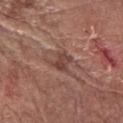Clinical impression:
This lesion was catalogued during total-body skin photography and was not selected for biopsy.
Clinical summary:
A male patient, in their mid- to late 70s. The lesion is on the right forearm. The recorded lesion diameter is about 3 mm. Imaged with white-light lighting. A 15 mm close-up extracted from a 3D total-body photography capture. An algorithmic analysis of the crop reported a footprint of about 5 mm², an eccentricity of roughly 0.55, and two-axis asymmetry of about 0.3. The analysis additionally found a border-irregularity rating of about 3.5/10 and radial color variation of about 1. The analysis additionally found a detector confidence of about 85 out of 100 that the crop contains a lesion.